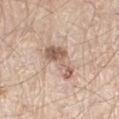This lesion was catalogued during total-body skin photography and was not selected for biopsy. Approximately 5.5 mm at its widest. Automated tile analysis of the lesion measured a lesion area of about 8 mm², a shape eccentricity near 0.95, and a shape-asymmetry score of about 0.6 (0 = symmetric). The software also gave about 13 CIELAB-L* units darker than the surrounding skin and a normalized lesion–skin contrast near 8.5. The software also gave a border-irregularity rating of about 7.5/10, internal color variation of about 4 on a 0–10 scale, and a peripheral color-asymmetry measure near 1. Located on the leg. Imaged with white-light lighting. A 15 mm close-up extracted from a 3D total-body photography capture. A male subject, roughly 60 years of age.